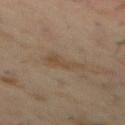A male patient about 55 years old. The tile uses cross-polarized illumination. Cropped from a total-body skin-imaging series; the visible field is about 15 mm. On the mid back. Approximately 4 mm at its widest. Automated image analysis of the tile measured a lesion area of about 6 mm² and a symmetry-axis asymmetry near 0.45. The analysis additionally found a mean CIELAB color near L≈45 a*≈13 b*≈28, roughly 6 lightness units darker than nearby skin, and a normalized border contrast of about 5.5. The software also gave a border-irregularity index near 5/10, a color-variation rating of about 2.5/10, and radial color variation of about 1.